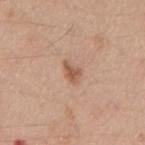<record>
  <biopsy_status>not biopsied; imaged during a skin examination</biopsy_status>
</record>